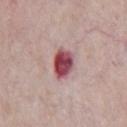Part of a total-body skin-imaging series; this lesion was reviewed on a skin check and was not flagged for biopsy. A male patient, aged 58–62. From the front of the torso. A 15 mm close-up extracted from a 3D total-body photography capture. Captured under white-light illumination.